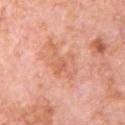  biopsy_status: not biopsied; imaged during a skin examination
  automated_metrics:
    area_mm2_approx: 8.0
    eccentricity: 0.85
    shape_asymmetry: 0.55
    cielab_L: 64
    cielab_a: 28
    cielab_b: 34
    vs_skin_contrast_norm: 5.0
    border_irregularity_0_10: 8.0
    color_variation_0_10: 3.5
    peripheral_color_asymmetry: 0.5
    nevus_likeness_0_100: 0
    lesion_detection_confidence_0_100: 100
  lesion_size:
    long_diameter_mm_approx: 5.0
  site: chest
  image:
    source: total-body photography crop
    field_of_view_mm: 15
  patient:
    sex: male
    age_approx: 80
  lighting: white-light